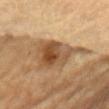Acquisition and patient details:
The lesion's longest dimension is about 7 mm. The lesion is on the mid back. This is a cross-polarized tile. An algorithmic analysis of the crop reported a footprint of about 19 mm², an eccentricity of roughly 0.75, and a shape-asymmetry score of about 0.4 (0 = symmetric). And it measured border irregularity of about 5 on a 0–10 scale and a color-variation rating of about 7/10. The software also gave an automated nevus-likeness rating near 85 out of 100. This image is a 15 mm lesion crop taken from a total-body photograph. A male patient, aged approximately 85 or older.